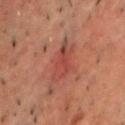Impression:
Part of a total-body skin-imaging series; this lesion was reviewed on a skin check and was not flagged for biopsy.
Image and clinical context:
The lesion is on the front of the torso. This image is a 15 mm lesion crop taken from a total-body photograph. The patient is a male roughly 50 years of age.